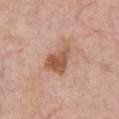notes = catalogued during a skin exam; not biopsied | subject = male, aged 58 to 62 | automated metrics = a footprint of about 9.5 mm², a shape eccentricity near 0.75, and two-axis asymmetry of about 0.4; roughly 11 lightness units darker than nearby skin and a normalized lesion–skin contrast near 8 | image source = ~15 mm crop, total-body skin-cancer survey | tile lighting = white-light illumination | location = the front of the torso.